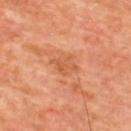The lesion was photographed on a routine skin check and not biopsied; there is no pathology result. The lesion is located on the upper back. A male patient, approximately 60 years of age. Captured under cross-polarized illumination. A lesion tile, about 15 mm wide, cut from a 3D total-body photograph. Longest diameter approximately 3.5 mm.